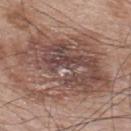A male subject, roughly 65 years of age.
From the upper back.
A 15 mm close-up extracted from a 3D total-body photography capture.
Approximately 10 mm at its widest.